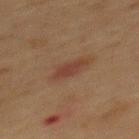{
  "biopsy_status": "not biopsied; imaged during a skin examination",
  "image": {
    "source": "total-body photography crop",
    "field_of_view_mm": 15
  },
  "patient": {
    "sex": "male",
    "age_approx": 50
  },
  "lighting": "cross-polarized",
  "automated_metrics": {
    "area_mm2_approx": 5.0,
    "eccentricity": 0.85,
    "cielab_L": 32,
    "cielab_a": 17,
    "cielab_b": 23,
    "vs_skin_darker_L": 6.0,
    "nevus_likeness_0_100": 95,
    "lesion_detection_confidence_0_100": 100
  },
  "lesion_size": {
    "long_diameter_mm_approx": 3.5
  },
  "site": "mid back"
}